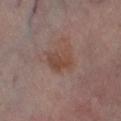Background:
From the left lower leg. A lesion tile, about 15 mm wide, cut from a 3D total-body photograph. A male patient aged 68–72. Longest diameter approximately 3 mm. Captured under cross-polarized illumination.